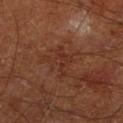The lesion was photographed on a routine skin check and not biopsied; there is no pathology result.
A region of skin cropped from a whole-body photographic capture, roughly 15 mm wide.
The patient is a male aged around 65.
From the right lower leg.